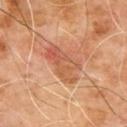Clinical summary:
Captured under cross-polarized illumination. Measured at roughly 5 mm in maximum diameter. A roughly 15 mm field-of-view crop from a total-body skin photograph. Located on the upper back. The patient is a male aged around 65.